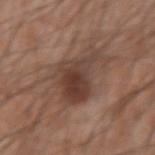– workup · total-body-photography surveillance lesion; no biopsy
– subject · male, aged around 30
– image · ~15 mm tile from a whole-body skin photo
– anatomic site · the left forearm